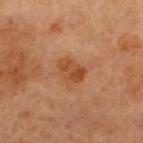location: the left lower leg | image: ~15 mm tile from a whole-body skin photo | patient: female, aged approximately 55 | automated lesion analysis: border irregularity of about 2.5 on a 0–10 scale, a within-lesion color-variation index near 4/10, and a peripheral color-asymmetry measure near 1.5; a classifier nevus-likeness of about 70/100 and a detector confidence of about 100 out of 100 that the crop contains a lesion | lesion size: ~3.5 mm (longest diameter) | lighting: cross-polarized.